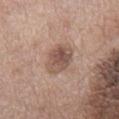– workup: imaged on a skin check; not biopsied
– imaging modality: ~15 mm crop, total-body skin-cancer survey
– body site: the chest
– subject: male, in their 70s